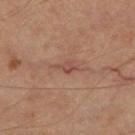The lesion was photographed on a routine skin check and not biopsied; there is no pathology result. A male subject, aged 63–67. This image is a 15 mm lesion crop taken from a total-body photograph. The lesion-visualizer software estimated a lesion color around L≈46 a*≈22 b*≈25 in CIELAB, about 7 CIELAB-L* units darker than the surrounding skin, and a lesion-to-skin contrast of about 6 (normalized; higher = more distinct). It also reported a border-irregularity rating of about 3.5/10, internal color variation of about 1 on a 0–10 scale, and a peripheral color-asymmetry measure near 0. It also reported lesion-presence confidence of about 95/100. From the right thigh. This is a cross-polarized tile. About 2.5 mm across.